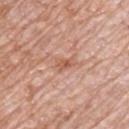<case>
  <biopsy_status>not biopsied; imaged during a skin examination</biopsy_status>
  <patient>
    <sex>male</sex>
    <age_approx>70</age_approx>
  </patient>
  <image>
    <source>total-body photography crop</source>
    <field_of_view_mm>15</field_of_view_mm>
  </image>
  <site>front of the torso</site>
</case>